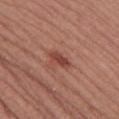<tbp_lesion>
<biopsy_status>not biopsied; imaged during a skin examination</biopsy_status>
<lighting>white-light</lighting>
<site>left thigh</site>
<image>
  <source>total-body photography crop</source>
  <field_of_view_mm>15</field_of_view_mm>
</image>
<patient>
  <sex>female</sex>
  <age_approx>30</age_approx>
</patient>
<lesion_size>
  <long_diameter_mm_approx>3.0</long_diameter_mm_approx>
</lesion_size>
</tbp_lesion>